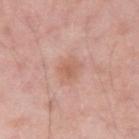Case summary:
* notes · catalogued during a skin exam; not biopsied
* anatomic site · the left upper arm
* tile lighting · white-light
* lesion size · ≈2.5 mm
* acquisition · ~15 mm crop, total-body skin-cancer survey
* patient · male, in their mid- to late 30s